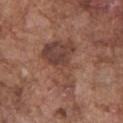Case summary:
• acquisition: total-body-photography crop, ~15 mm field of view
• location: the chest
• illumination: white-light illumination
• lesion size: ≈7.5 mm
• subject: male, approximately 75 years of age